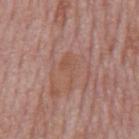biopsy_status: not biopsied; imaged during a skin examination
automated_metrics:
  area_mm2_approx: 5.5
  eccentricity: 0.9
  shape_asymmetry: 0.45
  cielab_L: 52
  cielab_a: 21
  cielab_b: 28
  border_irregularity_0_10: 5.5
  color_variation_0_10: 1.5
  peripheral_color_asymmetry: 0.5
  nevus_likeness_0_100: 0
  lesion_detection_confidence_0_100: 85
site: mid back
patient:
  sex: male
  age_approx: 75
lighting: white-light
lesion_size:
  long_diameter_mm_approx: 4.5
image:
  source: total-body photography crop
  field_of_view_mm: 15